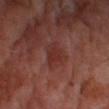Notes:
• workup · no biopsy performed (imaged during a skin exam)
• site · the leg
• image-analysis metrics · a footprint of about 4.5 mm², a shape eccentricity near 0.85, and a symmetry-axis asymmetry near 0.35; an average lesion color of about L≈30 a*≈25 b*≈23 (CIELAB) and a normalized lesion–skin contrast near 5.5; radial color variation of about 0.5; an automated nevus-likeness rating near 0 out of 100 and a lesion-detection confidence of about 80/100
• acquisition · ~15 mm tile from a whole-body skin photo
• lesion diameter · ≈3 mm
• subject · male, about 70 years old